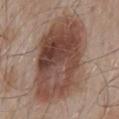| key | value |
|---|---|
| imaging modality | ~15 mm crop, total-body skin-cancer survey |
| patient | male, roughly 55 years of age |
| location | the mid back |